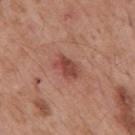| feature | finding |
|---|---|
| follow-up | imaged on a skin check; not biopsied |
| image source | total-body-photography crop, ~15 mm field of view |
| illumination | white-light |
| automated lesion analysis | a lesion color around L≈47 a*≈27 b*≈27 in CIELAB, roughly 10 lightness units darker than nearby skin, and a lesion-to-skin contrast of about 7.5 (normalized; higher = more distinct); an automated nevus-likeness rating near 40 out of 100 and a detector confidence of about 100 out of 100 that the crop contains a lesion |
| site | the mid back |
| lesion diameter | ~3.5 mm (longest diameter) |
| subject | male, in their mid-50s |